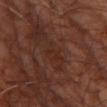Imaged during a routine full-body skin examination; the lesion was not biopsied and no histopathology is available. A male subject in their 60s. The recorded lesion diameter is about 2.5 mm. The lesion is on the leg. Imaged with cross-polarized lighting. A 15 mm close-up extracted from a 3D total-body photography capture. The total-body-photography lesion software estimated a border-irregularity rating of about 4/10, internal color variation of about 2 on a 0–10 scale, and peripheral color asymmetry of about 1.